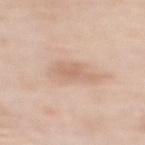{"biopsy_status": "not biopsied; imaged during a skin examination", "site": "upper back", "patient": {"sex": "female", "age_approx": 50}, "lighting": "white-light", "lesion_size": {"long_diameter_mm_approx": 3.5}, "image": {"source": "total-body photography crop", "field_of_view_mm": 15}}